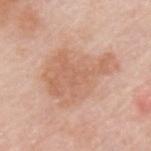notes = total-body-photography surveillance lesion; no biopsy | location = the upper back | subject = male, aged around 60 | image = total-body-photography crop, ~15 mm field of view.